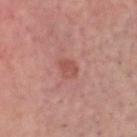The lesion was photographed on a routine skin check and not biopsied; there is no pathology result.
A male subject, aged 63–67.
Located on the head or neck.
A close-up tile cropped from a whole-body skin photograph, about 15 mm across.